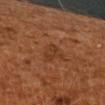Notes:
- notes — imaged on a skin check; not biopsied
- anatomic site — the left upper arm
- lesion diameter — ≈3 mm
- illumination — cross-polarized illumination
- imaging modality — ~15 mm tile from a whole-body skin photo
- patient — male, approximately 60 years of age